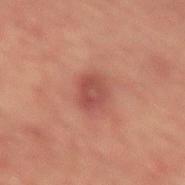{"biopsy_status": "not biopsied; imaged during a skin examination", "patient": {"sex": "male", "age_approx": 65}, "site": "lower back", "lighting": "cross-polarized", "lesion_size": {"long_diameter_mm_approx": 3.5}, "automated_metrics": {"area_mm2_approx": 7.5, "eccentricity": 0.6, "shape_asymmetry": 0.1, "cielab_L": 44, "cielab_a": 26, "cielab_b": 25, "vs_skin_darker_L": 8.0, "border_irregularity_0_10": 1.5, "color_variation_0_10": 4.0, "peripheral_color_asymmetry": 1.5}, "image": {"source": "total-body photography crop", "field_of_view_mm": 15}}